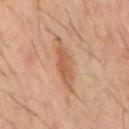Imaged during a routine full-body skin examination; the lesion was not biopsied and no histopathology is available.
A male subject, about 60 years old.
Cropped from a total-body skin-imaging series; the visible field is about 15 mm.
The lesion is on the back.